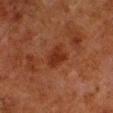lighting = cross-polarized | anatomic site = the left lower leg | automated metrics = a mean CIELAB color near L≈25 a*≈22 b*≈27 and roughly 7 lightness units darker than nearby skin; a border-irregularity rating of about 2.5/10, internal color variation of about 1.5 on a 0–10 scale, and a peripheral color-asymmetry measure near 0.5 | patient = male, aged around 80 | lesion size = about 3 mm | image source = ~15 mm tile from a whole-body skin photo.